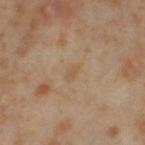Q: Was this lesion biopsied?
A: no biopsy performed (imaged during a skin exam)
Q: What are the patient's age and sex?
A: female, aged approximately 55
Q: Lesion size?
A: ≈2.5 mm
Q: Automated lesion metrics?
A: a footprint of about 3 mm², an outline eccentricity of about 0.85 (0 = round, 1 = elongated), and a shape-asymmetry score of about 0.25 (0 = symmetric); an average lesion color of about L≈56 a*≈14 b*≈32 (CIELAB), about 5 CIELAB-L* units darker than the surrounding skin, and a normalized border contrast of about 4.5; border irregularity of about 2.5 on a 0–10 scale, a color-variation rating of about 0.5/10, and a peripheral color-asymmetry measure near 0.5
Q: What lighting was used for the tile?
A: cross-polarized
Q: What is the anatomic site?
A: the left thigh
Q: How was this image acquired?
A: ~15 mm crop, total-body skin-cancer survey